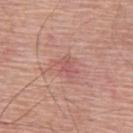The lesion was photographed on a routine skin check and not biopsied; there is no pathology result. Automated tile analysis of the lesion measured an area of roughly 2.5 mm², an outline eccentricity of about 0.85 (0 = round, 1 = elongated), and a symmetry-axis asymmetry near 0.35. The software also gave a border-irregularity rating of about 3.5/10, internal color variation of about 0 on a 0–10 scale, and peripheral color asymmetry of about 0. A roughly 15 mm field-of-view crop from a total-body skin photograph. From the upper back. Longest diameter approximately 2.5 mm. A male subject aged 58–62.